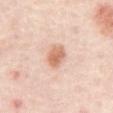biopsy status: total-body-photography surveillance lesion; no biopsy
location: the abdomen
patient: aged around 55
diameter: ≈3.5 mm
image source: ~15 mm tile from a whole-body skin photo